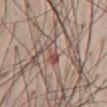Part of a total-body skin-imaging series; this lesion was reviewed on a skin check and was not flagged for biopsy. Imaged with white-light lighting. The lesion is located on the abdomen. Cropped from a whole-body photographic skin survey; the tile spans about 15 mm. A male subject about 60 years old. The lesion's longest dimension is about 3 mm. The lesion-visualizer software estimated a lesion area of about 4 mm², a shape eccentricity near 0.85, and two-axis asymmetry of about 0.25. It also reported a lesion color around L≈51 a*≈20 b*≈23 in CIELAB and about 8 CIELAB-L* units darker than the surrounding skin. And it measured a classifier nevus-likeness of about 0/100 and lesion-presence confidence of about 50/100.